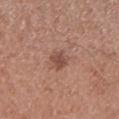Case summary:
• follow-up — no biopsy performed (imaged during a skin exam)
• location — the left lower leg
• image source — total-body-photography crop, ~15 mm field of view
• automated lesion analysis — a footprint of about 4.5 mm², an eccentricity of roughly 0.55, and two-axis asymmetry of about 0.2; an automated nevus-likeness rating near 60 out of 100 and a lesion-detection confidence of about 100/100
• subject — male, about 50 years old
• diameter — ≈2.5 mm
• illumination — white-light illumination